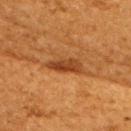Part of a total-body skin-imaging series; this lesion was reviewed on a skin check and was not flagged for biopsy. A lesion tile, about 15 mm wide, cut from a 3D total-body photograph. This is a cross-polarized tile. Longest diameter approximately 4.5 mm. The lesion is on the upper back. A female subject, aged 48–52.